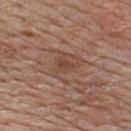Clinical impression: The lesion was tiled from a total-body skin photograph and was not biopsied. Context: The lesion's longest dimension is about 2.5 mm. A roughly 15 mm field-of-view crop from a total-body skin photograph. The lesion is located on the back. Captured under white-light illumination. The subject is a male roughly 80 years of age.